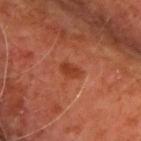Notes:
- biopsy status · catalogued during a skin exam; not biopsied
- body site · the upper back
- size · ~2.5 mm (longest diameter)
- imaging modality · total-body-photography crop, ~15 mm field of view
- illumination · cross-polarized illumination
- subject · male, aged 63–67